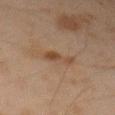{"biopsy_status": "not biopsied; imaged during a skin examination", "image": {"source": "total-body photography crop", "field_of_view_mm": 15}, "lesion_size": {"long_diameter_mm_approx": 3.0}, "automated_metrics": {"peripheral_color_asymmetry": 1.0}, "lighting": "cross-polarized", "site": "left forearm", "patient": {"sex": "male", "age_approx": 45}}